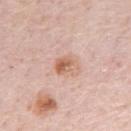Q: Is there a histopathology result?
A: catalogued during a skin exam; not biopsied
Q: What is the anatomic site?
A: the abdomen
Q: What is the imaging modality?
A: 15 mm crop, total-body photography
Q: What are the patient's age and sex?
A: male, aged 78 to 82